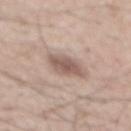– notes: total-body-photography surveillance lesion; no biopsy
– subject: male, roughly 55 years of age
– automated metrics: an area of roughly 7.5 mm², an eccentricity of roughly 0.75, and two-axis asymmetry of about 0.2
– lesion diameter: about 4 mm
– location: the mid back
– image source: 15 mm crop, total-body photography